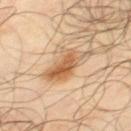Part of a total-body skin-imaging series; this lesion was reviewed on a skin check and was not flagged for biopsy. A 15 mm close-up tile from a total-body photography series done for melanoma screening. A male subject approximately 65 years of age. Located on the right thigh.